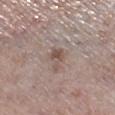This lesion was catalogued during total-body skin photography and was not selected for biopsy. A female patient, in their mid- to late 60s. From the right lower leg. Cropped from a total-body skin-imaging series; the visible field is about 15 mm.